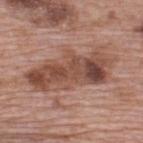Findings:
– biopsy status — catalogued during a skin exam; not biopsied
– size — ~10 mm (longest diameter)
– tile lighting — white-light illumination
– anatomic site — the upper back
– TBP lesion metrics — an area of roughly 29 mm² and a shape-asymmetry score of about 0.25 (0 = symmetric); a classifier nevus-likeness of about 10/100 and a detector confidence of about 100 out of 100 that the crop contains a lesion
– patient — male, aged 68 to 72
– acquisition — ~15 mm tile from a whole-body skin photo